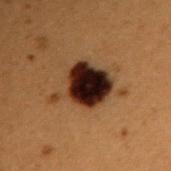The lesion was photographed on a routine skin check and not biopsied; there is no pathology result. The total-body-photography lesion software estimated a classifier nevus-likeness of about 0/100 and lesion-presence confidence of about 100/100. On the right upper arm. A male patient, about 50 years old. Cropped from a whole-body photographic skin survey; the tile spans about 15 mm. The lesion's longest dimension is about 5.5 mm.